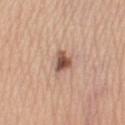Assessment:
The lesion was tiled from a total-body skin photograph and was not biopsied.
Background:
A female subject approximately 60 years of age. The lesion's longest dimension is about 3.5 mm. Captured under white-light illumination. Cropped from a whole-body photographic skin survey; the tile spans about 15 mm. The lesion is located on the leg. The lesion-visualizer software estimated a color-variation rating of about 4/10 and radial color variation of about 1.5. It also reported a detector confidence of about 100 out of 100 that the crop contains a lesion.